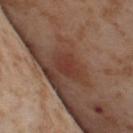| key | value |
|---|---|
| follow-up | imaged on a skin check; not biopsied |
| imaging modality | total-body-photography crop, ~15 mm field of view |
| patient | female, aged 53–57 |
| lighting | cross-polarized |
| automated metrics | an eccentricity of roughly 0.75 and two-axis asymmetry of about 0.3; a nevus-likeness score of about 20/100 and a lesion-detection confidence of about 90/100 |
| location | the left thigh |